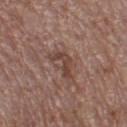{"biopsy_status": "not biopsied; imaged during a skin examination", "lighting": "white-light", "image": {"source": "total-body photography crop", "field_of_view_mm": 15}, "site": "left thigh", "lesion_size": {"long_diameter_mm_approx": 3.5}, "patient": {"sex": "male", "age_approx": 70}}